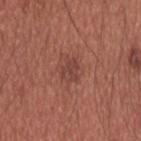  biopsy_status: not biopsied; imaged during a skin examination
  patient:
    sex: male
    age_approx: 35
  image:
    source: total-body photography crop
    field_of_view_mm: 15
  automated_metrics:
    cielab_L: 43
    cielab_a: 24
    cielab_b: 25
    vs_skin_darker_L: 7.0
    vs_skin_contrast_norm: 6.0
  lighting: white-light
  site: upper back
  lesion_size:
    long_diameter_mm_approx: 2.5